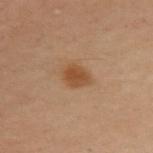Q: What is the imaging modality?
A: ~15 mm crop, total-body skin-cancer survey
Q: What is the lesion's diameter?
A: ~2.5 mm (longest diameter)
Q: What did automated image analysis measure?
A: an average lesion color of about L≈41 a*≈18 b*≈31 (CIELAB), a lesion–skin lightness drop of about 8, and a normalized border contrast of about 8
Q: Where on the body is the lesion?
A: the arm
Q: Patient demographics?
A: female, aged around 50
Q: Illumination type?
A: cross-polarized